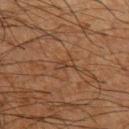Recorded during total-body skin imaging; not selected for excision or biopsy. Measured at roughly 2.5 mm in maximum diameter. The lesion is on the left upper arm. Cropped from a total-body skin-imaging series; the visible field is about 15 mm. The lesion-visualizer software estimated a footprint of about 2.5 mm², a shape eccentricity near 0.85, and a symmetry-axis asymmetry near 0.4. It also reported a mean CIELAB color near L≈32 a*≈16 b*≈26, a lesion–skin lightness drop of about 5, and a normalized lesion–skin contrast near 5. The software also gave border irregularity of about 4.5 on a 0–10 scale, a color-variation rating of about 0/10, and radial color variation of about 0. And it measured an automated nevus-likeness rating near 0 out of 100 and lesion-presence confidence of about 60/100. A male subject about 65 years old. Captured under cross-polarized illumination.